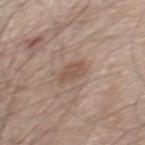notes: imaged on a skin check; not biopsied | image-analysis metrics: a mean CIELAB color near L≈53 a*≈17 b*≈26, roughly 9 lightness units darker than nearby skin, and a lesion-to-skin contrast of about 6.5 (normalized; higher = more distinct); border irregularity of about 2 on a 0–10 scale, a within-lesion color-variation index near 2/10, and peripheral color asymmetry of about 0.5 | lesion diameter: ~3.5 mm (longest diameter) | body site: the mid back | subject: male, aged 48 to 52 | image: ~15 mm crop, total-body skin-cancer survey.